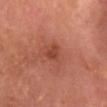Imaged during a routine full-body skin examination; the lesion was not biopsied and no histopathology is available.